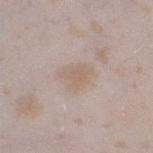Clinical impression: Imaged during a routine full-body skin examination; the lesion was not biopsied and no histopathology is available. Clinical summary: On the right thigh. The lesion's longest dimension is about 3.5 mm. The patient is a female in their mid- to late 20s. A 15 mm close-up tile from a total-body photography series done for melanoma screening. The tile uses white-light illumination.